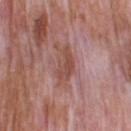Impression:
Imaged during a routine full-body skin examination; the lesion was not biopsied and no histopathology is available.
Context:
The lesion's longest dimension is about 4.5 mm. Imaged with white-light lighting. From the back. A male patient about 60 years old. A region of skin cropped from a whole-body photographic capture, roughly 15 mm wide.